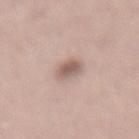{"biopsy_status": "not biopsied; imaged during a skin examination", "lighting": "white-light", "image": {"source": "total-body photography crop", "field_of_view_mm": 15}, "lesion_size": {"long_diameter_mm_approx": 3.0}, "automated_metrics": {"vs_skin_darker_L": 12.0, "vs_skin_contrast_norm": 7.5}, "patient": {"sex": "female", "age_approx": 50}, "site": "lower back"}